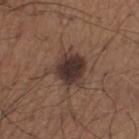Findings:
– biopsy status · total-body-photography surveillance lesion; no biopsy